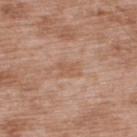Q: Is there a histopathology result?
A: no biopsy performed (imaged during a skin exam)
Q: Lesion location?
A: the upper back
Q: Illumination type?
A: white-light
Q: Who is the patient?
A: male, approximately 50 years of age
Q: How was this image acquired?
A: 15 mm crop, total-body photography